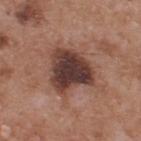| feature | finding |
|---|---|
| notes | no biopsy performed (imaged during a skin exam) |
| acquisition | ~15 mm tile from a whole-body skin photo |
| site | the back |
| subject | male, in their mid- to late 50s |